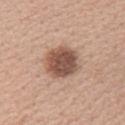Clinical summary: The lesion is located on the upper back. A female patient aged approximately 40. The total-body-photography lesion software estimated an area of roughly 13 mm², an outline eccentricity of about 0.4 (0 = round, 1 = elongated), and two-axis asymmetry of about 0.1. The software also gave a mean CIELAB color near L≈51 a*≈20 b*≈27 and a normalized border contrast of about 10.5. And it measured a nevus-likeness score of about 40/100. Cropped from a total-body skin-imaging series; the visible field is about 15 mm.